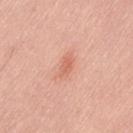Case summary:
- workup · catalogued during a skin exam; not biopsied
- lesion diameter · ≈2.5 mm
- patient · male, in their mid-50s
- lighting · white-light
- acquisition · ~15 mm tile from a whole-body skin photo
- automated metrics · a border-irregularity index near 3/10, internal color variation of about 1 on a 0–10 scale, and radial color variation of about 0
- site · the back